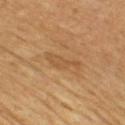- workup: no biopsy performed (imaged during a skin exam)
- patient: male, aged 58–62
- acquisition: total-body-photography crop, ~15 mm field of view
- body site: the chest
- lighting: cross-polarized illumination